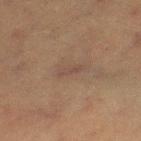The lesion was tiled from a total-body skin photograph and was not biopsied. An algorithmic analysis of the crop reported an outline eccentricity of about 0.95 (0 = round, 1 = elongated) and two-axis asymmetry of about 0.4. The patient is a female aged around 55. A 15 mm close-up tile from a total-body photography series done for melanoma screening. The lesion's longest dimension is about 3 mm. The lesion is located on the left thigh.